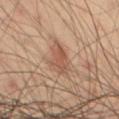Captured during whole-body skin photography for melanoma surveillance; the lesion was not biopsied. On the leg. A male patient, in their 40s. Longest diameter approximately 4.5 mm. A region of skin cropped from a whole-body photographic capture, roughly 15 mm wide.